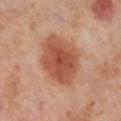| field | value |
|---|---|
| workup | no biopsy performed (imaged during a skin exam) |
| image-analysis metrics | an eccentricity of roughly 0.65 and a symmetry-axis asymmetry near 0.15; a border-irregularity rating of about 1.5/10, internal color variation of about 4.5 on a 0–10 scale, and a peripheral color-asymmetry measure near 1; a nevus-likeness score of about 95/100 and lesion-presence confidence of about 100/100 |
| site | the right lower leg |
| size | about 6 mm |
| patient | female, aged around 55 |
| image source | 15 mm crop, total-body photography |